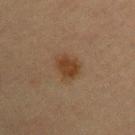Imaged during a routine full-body skin examination; the lesion was not biopsied and no histopathology is available. The lesion-visualizer software estimated a footprint of about 7 mm² and a symmetry-axis asymmetry near 0.3. The analysis additionally found a lesion-to-skin contrast of about 8.5 (normalized; higher = more distinct). It also reported a nevus-likeness score of about 100/100 and lesion-presence confidence of about 100/100. A close-up tile cropped from a whole-body skin photograph, about 15 mm across. A female patient, aged 63–67. The recorded lesion diameter is about 3 mm. On the arm.